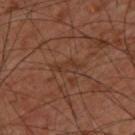Q: Is there a histopathology result?
A: imaged on a skin check; not biopsied
Q: What lighting was used for the tile?
A: cross-polarized illumination
Q: How large is the lesion?
A: ~3.5 mm (longest diameter)
Q: What kind of image is this?
A: total-body-photography crop, ~15 mm field of view
Q: Who is the patient?
A: male, approximately 60 years of age
Q: What is the anatomic site?
A: the back